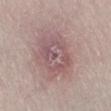This lesion was catalogued during total-body skin photography and was not selected for biopsy.
A lesion tile, about 15 mm wide, cut from a 3D total-body photograph.
The lesion is located on the right lower leg.
A female patient aged 58–62.